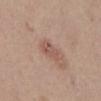Recorded during total-body skin imaging; not selected for excision or biopsy.
The tile uses white-light illumination.
The patient is a female aged 38 to 42.
The total-body-photography lesion software estimated a lesion area of about 5.5 mm², an eccentricity of roughly 0.8, and a shape-asymmetry score of about 0.3 (0 = symmetric). And it measured a lesion color around L≈54 a*≈20 b*≈26 in CIELAB, about 8 CIELAB-L* units darker than the surrounding skin, and a normalized lesion–skin contrast near 5.5. And it measured a border-irregularity rating of about 3/10 and radial color variation of about 1.
Cropped from a whole-body photographic skin survey; the tile spans about 15 mm.
From the abdomen.